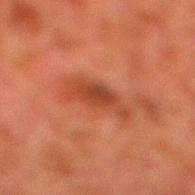follow-up: imaged on a skin check; not biopsied
imaging modality: ~15 mm tile from a whole-body skin photo
automated metrics: a lesion area of about 9 mm², an outline eccentricity of about 0.9 (0 = round, 1 = elongated), and a shape-asymmetry score of about 0.4 (0 = symmetric); a lesion color around L≈36 a*≈26 b*≈30 in CIELAB, about 8 CIELAB-L* units darker than the surrounding skin, and a lesion-to-skin contrast of about 7 (normalized; higher = more distinct); a border-irregularity rating of about 4.5/10, a within-lesion color-variation index near 3.5/10, and peripheral color asymmetry of about 0.5
illumination: cross-polarized illumination
location: the right lower leg
diameter: about 5 mm
subject: male, aged around 80